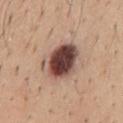workup: no biopsy performed (imaged during a skin exam).